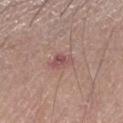Q: Is there a histopathology result?
A: total-body-photography surveillance lesion; no biopsy
Q: What is the lesion's diameter?
A: ≈2.5 mm
Q: How was this image acquired?
A: ~15 mm crop, total-body skin-cancer survey
Q: Automated lesion metrics?
A: a footprint of about 4 mm² and a shape eccentricity near 0.75; an average lesion color of about L≈51 a*≈23 b*≈21 (CIELAB), a lesion–skin lightness drop of about 9, and a normalized border contrast of about 6.5; a border-irregularity rating of about 2/10, internal color variation of about 4 on a 0–10 scale, and a peripheral color-asymmetry measure near 1.5; a classifier nevus-likeness of about 0/100 and lesion-presence confidence of about 100/100
Q: Who is the patient?
A: male, about 65 years old
Q: Illumination type?
A: white-light
Q: Where on the body is the lesion?
A: the right forearm